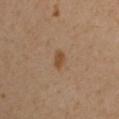follow-up: imaged on a skin check; not biopsied | size: ~2.5 mm (longest diameter) | site: the right upper arm | illumination: cross-polarized illumination | patient: male, in their mid- to late 40s | image: total-body-photography crop, ~15 mm field of view | image-analysis metrics: a border-irregularity index near 2.5/10 and a color-variation rating of about 1/10; an automated nevus-likeness rating near 85 out of 100 and lesion-presence confidence of about 100/100.